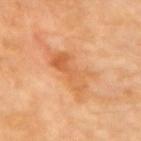Assessment: Captured during whole-body skin photography for melanoma surveillance; the lesion was not biopsied. Image and clinical context: A 15 mm close-up extracted from a 3D total-body photography capture. Captured under cross-polarized illumination. Located on the arm. The subject is a female in their 70s. The lesion-visualizer software estimated an area of roughly 14 mm², an outline eccentricity of about 0.85 (0 = round, 1 = elongated), and a symmetry-axis asymmetry near 0.45. The analysis additionally found about 9 CIELAB-L* units darker than the surrounding skin and a normalized lesion–skin contrast near 6. The software also gave a border-irregularity index near 7/10, a color-variation rating of about 6.5/10, and a peripheral color-asymmetry measure near 2.